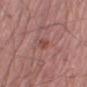Part of a total-body skin-imaging series; this lesion was reviewed on a skin check and was not flagged for biopsy. The tile uses white-light illumination. The subject is a male aged approximately 60. Automated tile analysis of the lesion measured an area of roughly 2.5 mm², a shape eccentricity near 0.9, and a shape-asymmetry score of about 0.3 (0 = symmetric). The software also gave a lesion color around L≈46 a*≈24 b*≈23 in CIELAB and a lesion–skin lightness drop of about 7. The analysis additionally found border irregularity of about 3 on a 0–10 scale, a within-lesion color-variation index near 0/10, and radial color variation of about 0. The lesion is located on the right lower leg. A 15 mm close-up tile from a total-body photography series done for melanoma screening. Approximately 2.5 mm at its widest.